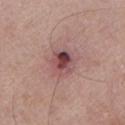Clinical impression:
This lesion was catalogued during total-body skin photography and was not selected for biopsy.
Background:
The recorded lesion diameter is about 3 mm. Automated tile analysis of the lesion measured a lesion color around L≈48 a*≈23 b*≈19 in CIELAB and about 12 CIELAB-L* units darker than the surrounding skin. It also reported a border-irregularity rating of about 1.5/10, a color-variation rating of about 10/10, and peripheral color asymmetry of about 4. It also reported an automated nevus-likeness rating near 15 out of 100 and a lesion-detection confidence of about 100/100. Cropped from a total-body skin-imaging series; the visible field is about 15 mm. The lesion is located on the left lower leg. A male patient, approximately 80 years of age.